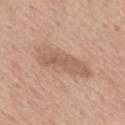Q: Was a biopsy performed?
A: imaged on a skin check; not biopsied
Q: Automated lesion metrics?
A: a shape eccentricity near 0.95 and a shape-asymmetry score of about 0.25 (0 = symmetric); an automated nevus-likeness rating near 0 out of 100 and a lesion-detection confidence of about 100/100
Q: How was the tile lit?
A: white-light illumination
Q: Lesion location?
A: the mid back
Q: Who is the patient?
A: male, approximately 60 years of age
Q: What is the imaging modality?
A: ~15 mm tile from a whole-body skin photo
Q: How large is the lesion?
A: about 7.5 mm